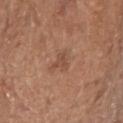biopsy_status: not biopsied; imaged during a skin examination
site: head or neck
image:
  source: total-body photography crop
  field_of_view_mm: 15
lighting: white-light
patient:
  sex: male
  age_approx: 80
lesion_size:
  long_diameter_mm_approx: 3.0